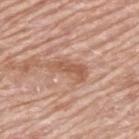Notes:
- notes: catalogued during a skin exam; not biopsied
- automated metrics: a lesion area of about 6.5 mm², a shape eccentricity near 0.95, and a shape-asymmetry score of about 0.35 (0 = symmetric); a lesion-detection confidence of about 95/100
- imaging modality: ~15 mm tile from a whole-body skin photo
- lighting: white-light illumination
- patient: male, aged around 80
- body site: the upper back